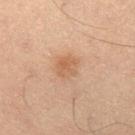{"biopsy_status": "not biopsied; imaged during a skin examination", "lesion_size": {"long_diameter_mm_approx": 3.0}, "site": "right thigh", "image": {"source": "total-body photography crop", "field_of_view_mm": 15}, "patient": {"sex": "male", "age_approx": 65}}